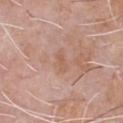Q: What is the lesion's diameter?
A: ≈2.5 mm
Q: Lesion location?
A: the front of the torso
Q: What kind of image is this?
A: 15 mm crop, total-body photography
Q: What are the patient's age and sex?
A: male, aged 58 to 62